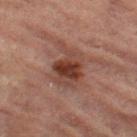No biopsy was performed on this lesion — it was imaged during a full skin examination and was not determined to be concerning.
The lesion is located on the left thigh.
The patient is a female aged 68 to 72.
The tile uses cross-polarized illumination.
Longest diameter approximately 5 mm.
A region of skin cropped from a whole-body photographic capture, roughly 15 mm wide.